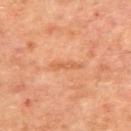follow-up=catalogued during a skin exam; not biopsied
subject=male, about 65 years old
image=~15 mm tile from a whole-body skin photo
illumination=cross-polarized
location=the upper back